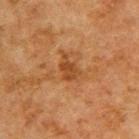Assessment:
Captured during whole-body skin photography for melanoma surveillance; the lesion was not biopsied.
Image and clinical context:
Captured under cross-polarized illumination. On the upper back. An algorithmic analysis of the crop reported a footprint of about 4.5 mm², a shape eccentricity near 0.75, and a symmetry-axis asymmetry near 0.4. The analysis additionally found a lesion color around L≈37 a*≈21 b*≈34 in CIELAB and about 8 CIELAB-L* units darker than the surrounding skin. The software also gave a border-irregularity index near 4/10, a within-lesion color-variation index near 2/10, and a peripheral color-asymmetry measure near 0.5. A 15 mm close-up tile from a total-body photography series done for melanoma screening. Measured at roughly 3 mm in maximum diameter. A male subject roughly 50 years of age.